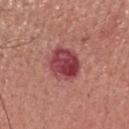Part of a total-body skin-imaging series; this lesion was reviewed on a skin check and was not flagged for biopsy.
A region of skin cropped from a whole-body photographic capture, roughly 15 mm wide.
The lesion is located on the head or neck.
Longest diameter approximately 4.5 mm.
A female patient aged approximately 65.
This is a white-light tile.